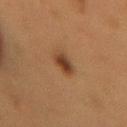biopsy_status: not biopsied; imaged during a skin examination
patient:
  sex: female
  age_approx: 50
lesion_size:
  long_diameter_mm_approx: 3.0
lighting: cross-polarized
image:
  source: total-body photography crop
  field_of_view_mm: 15
site: back